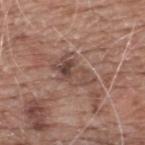The total-body-photography lesion software estimated a lesion area of about 10 mm², an eccentricity of roughly 0.8, and a symmetry-axis asymmetry near 0.3. The software also gave a lesion color around L≈47 a*≈18 b*≈24 in CIELAB, a lesion–skin lightness drop of about 9, and a normalized lesion–skin contrast near 6.5. The software also gave internal color variation of about 8 on a 0–10 scale and peripheral color asymmetry of about 3. About 5 mm across. On the back. A 15 mm close-up tile from a total-body photography series done for melanoma screening. The patient is a male in their 60s. Imaged with white-light lighting.